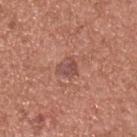Findings:
• follow-up: imaged on a skin check; not biopsied
• image: ~15 mm tile from a whole-body skin photo
• automated lesion analysis: a lesion area of about 4 mm², an eccentricity of roughly 0.6, and a symmetry-axis asymmetry near 0.35; a mean CIELAB color near L≈50 a*≈24 b*≈25; a nevus-likeness score of about 0/100
• site: the upper back
• size: ~2.5 mm (longest diameter)
• subject: male, aged 53 to 57